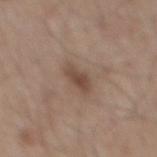Q: Was a biopsy performed?
A: no biopsy performed (imaged during a skin exam)
Q: Lesion location?
A: the mid back
Q: What did automated image analysis measure?
A: an area of roughly 4.5 mm²; a lesion color around L≈46 a*≈17 b*≈25 in CIELAB and a lesion-to-skin contrast of about 7.5 (normalized; higher = more distinct); border irregularity of about 2.5 on a 0–10 scale, a color-variation rating of about 2.5/10, and a peripheral color-asymmetry measure near 1; an automated nevus-likeness rating near 70 out of 100 and lesion-presence confidence of about 100/100
Q: What are the patient's age and sex?
A: male, aged 73 to 77
Q: How was this image acquired?
A: ~15 mm tile from a whole-body skin photo
Q: What is the lesion's diameter?
A: ≈2.5 mm